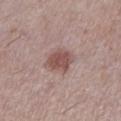Assessment: This lesion was catalogued during total-body skin photography and was not selected for biopsy. Background: On the left lower leg. A female patient aged approximately 60. This image is a 15 mm lesion crop taken from a total-body photograph. Captured under white-light illumination.